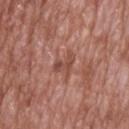Imaged during a routine full-body skin examination; the lesion was not biopsied and no histopathology is available.
The lesion's longest dimension is about 3 mm.
The subject is a male about 70 years old.
The tile uses white-light illumination.
This image is a 15 mm lesion crop taken from a total-body photograph.
The lesion is located on the upper back.